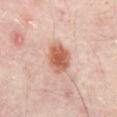<tbp_lesion>
  <biopsy_status>not biopsied; imaged during a skin examination</biopsy_status>
  <image>
    <source>total-body photography crop</source>
    <field_of_view_mm>15</field_of_view_mm>
  </image>
  <lesion_size>
    <long_diameter_mm_approx>4.0</long_diameter_mm_approx>
  </lesion_size>
  <automated_metrics>
    <shape_asymmetry>0.1</shape_asymmetry>
  </automated_metrics>
  <site>abdomen</site>
  <patient>
    <age_approx>55</age_approx>
  </patient>
</tbp_lesion>